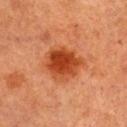No biopsy was performed on this lesion — it was imaged during a full skin examination and was not determined to be concerning. Imaged with cross-polarized lighting. Measured at roughly 4.5 mm in maximum diameter. On the chest. The patient is a female aged 58–62. A 15 mm close-up tile from a total-body photography series done for melanoma screening.